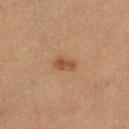The total-body-photography lesion software estimated an average lesion color of about L≈50 a*≈22 b*≈34 (CIELAB) and about 9 CIELAB-L* units darker than the surrounding skin. And it measured internal color variation of about 3 on a 0–10 scale and peripheral color asymmetry of about 1. The analysis additionally found an automated nevus-likeness rating near 85 out of 100.
Approximately 2.5 mm at its widest.
A female subject roughly 50 years of age.
Imaged with cross-polarized lighting.
From the left lower leg.
This image is a 15 mm lesion crop taken from a total-body photograph.